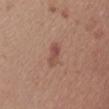biopsy_status: not biopsied; imaged during a skin examination
patient:
  sex: female
  age_approx: 55
automated_metrics:
  border_irregularity_0_10: 3.5
  color_variation_0_10: 7.0
  nevus_likeness_0_100: 40
lighting: white-light
image:
  source: total-body photography crop
  field_of_view_mm: 15
site: front of the torso
lesion_size:
  long_diameter_mm_approx: 3.5